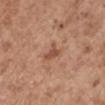Clinical impression:
The lesion was photographed on a routine skin check and not biopsied; there is no pathology result.
Acquisition and patient details:
A 15 mm crop from a total-body photograph taken for skin-cancer surveillance. Located on the right upper arm. A female patient aged 63 to 67.